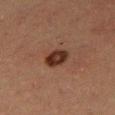Part of a total-body skin-imaging series; this lesion was reviewed on a skin check and was not flagged for biopsy. The subject is a female approximately 40 years of age. Cropped from a whole-body photographic skin survey; the tile spans about 15 mm. Located on the right thigh. Imaged with cross-polarized lighting. Automated image analysis of the tile measured an outline eccentricity of about 0.6 (0 = round, 1 = elongated) and a shape-asymmetry score of about 0.15 (0 = symmetric).